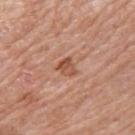Impression:
No biopsy was performed on this lesion — it was imaged during a full skin examination and was not determined to be concerning.
Image and clinical context:
The tile uses white-light illumination. Cropped from a whole-body photographic skin survey; the tile spans about 15 mm. The lesion is located on the right upper arm. The subject is a male aged around 60. About 2.5 mm across.